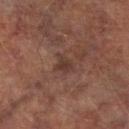{"biopsy_status": "not biopsied; imaged during a skin examination", "automated_metrics": {"area_mm2_approx": 4.0, "eccentricity": 0.55, "shape_asymmetry": 0.35, "border_irregularity_0_10": 3.5, "color_variation_0_10": 1.5, "peripheral_color_asymmetry": 0.5}, "patient": {"sex": "male", "age_approx": 75}, "image": {"source": "total-body photography crop", "field_of_view_mm": 15}, "lighting": "cross-polarized", "site": "right lower leg", "lesion_size": {"long_diameter_mm_approx": 2.5}}